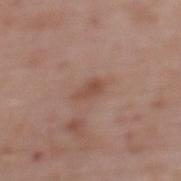Impression:
Imaged during a routine full-body skin examination; the lesion was not biopsied and no histopathology is available.
Context:
A 15 mm close-up tile from a total-body photography series done for melanoma screening. The lesion is located on the upper back. Imaged with white-light lighting. The total-body-photography lesion software estimated an area of roughly 3 mm² and a shape-asymmetry score of about 0.3 (0 = symmetric). It also reported a border-irregularity rating of about 3.5/10 and a within-lesion color-variation index near 1/10. A female patient about 65 years old.